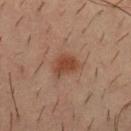Impression: The lesion was tiled from a total-body skin photograph and was not biopsied. Image and clinical context: Located on the front of the torso. A lesion tile, about 15 mm wide, cut from a 3D total-body photograph. Imaged with cross-polarized lighting. The subject is a male in their mid-30s. Automated image analysis of the tile measured a lesion–skin lightness drop of about 8 and a normalized lesion–skin contrast near 7.5.